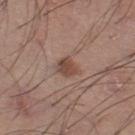follow-up: total-body-photography surveillance lesion; no biopsy
patient: male, about 55 years old
acquisition: ~15 mm crop, total-body skin-cancer survey
anatomic site: the right thigh